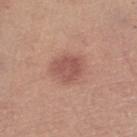  biopsy_status: not biopsied; imaged during a skin examination
  lighting: white-light
  patient:
    sex: female
    age_approx: 65
  lesion_size:
    long_diameter_mm_approx: 3.5
  image:
    source: total-body photography crop
    field_of_view_mm: 15
  site: left lower leg
  automated_metrics:
    cielab_L: 53
    cielab_a: 24
    cielab_b: 25
    vs_skin_darker_L: 10.0
    vs_skin_contrast_norm: 7.0
    border_irregularity_0_10: 2.0
    color_variation_0_10: 2.5
    peripheral_color_asymmetry: 1.0